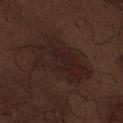Recorded during total-body skin imaging; not selected for excision or biopsy.
The lesion is on the abdomen.
Cropped from a whole-body photographic skin survey; the tile spans about 15 mm.
A male subject, aged approximately 70.
Imaged with white-light lighting.
The lesion's longest dimension is about 7.5 mm.